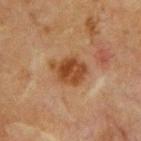{"biopsy_status": "not biopsied; imaged during a skin examination", "lesion_size": {"long_diameter_mm_approx": 4.5}, "site": "chest", "image": {"source": "total-body photography crop", "field_of_view_mm": 15}, "patient": {"sex": "male", "age_approx": 65}, "lighting": "cross-polarized"}